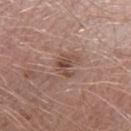* follow-up — total-body-photography surveillance lesion; no biopsy
* image — total-body-photography crop, ~15 mm field of view
* TBP lesion metrics — a peripheral color-asymmetry measure near 1; an automated nevus-likeness rating near 5 out of 100
* body site — the right forearm
* patient — male, aged 48–52